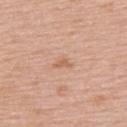Imaged during a routine full-body skin examination; the lesion was not biopsied and no histopathology is available. A 15 mm crop from a total-body photograph taken for skin-cancer surveillance. About 2.5 mm across. The subject is a male roughly 55 years of age. Captured under white-light illumination. The lesion is located on the upper back.